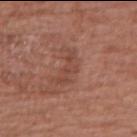<record>
  <biopsy_status>not biopsied; imaged during a skin examination</biopsy_status>
  <image>
    <source>total-body photography crop</source>
    <field_of_view_mm>15</field_of_view_mm>
  </image>
  <site>upper back</site>
  <patient>
    <sex>female</sex>
    <age_approx>80</age_approx>
  </patient>
</record>